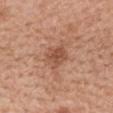biopsy status = no biopsy performed (imaged during a skin exam); image = ~15 mm crop, total-body skin-cancer survey; location = the back; subject = female, aged 38 to 42.